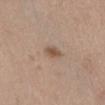Q: Was this lesion biopsied?
A: total-body-photography surveillance lesion; no biopsy
Q: What are the patient's age and sex?
A: female, aged 38 to 42
Q: What lighting was used for the tile?
A: white-light illumination
Q: What is the imaging modality?
A: ~15 mm crop, total-body skin-cancer survey
Q: What did automated image analysis measure?
A: roughly 10 lightness units darker than nearby skin and a normalized border contrast of about 7.5; a border-irregularity rating of about 2.5/10, a color-variation rating of about 1.5/10, and radial color variation of about 0.5; a classifier nevus-likeness of about 70/100 and a lesion-detection confidence of about 100/100
Q: What is the lesion's diameter?
A: about 2.5 mm
Q: Where on the body is the lesion?
A: the front of the torso